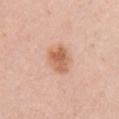Case summary:
* workup: catalogued during a skin exam; not biopsied
* lesion size: ~3.5 mm (longest diameter)
* site: the front of the torso
* patient: female, aged 28 to 32
* imaging modality: ~15 mm crop, total-body skin-cancer survey
* illumination: white-light illumination